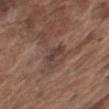notes — no biopsy performed (imaged during a skin exam) | diameter — ≈3 mm | subject — male, in their mid- to late 70s | tile lighting — white-light illumination | image source — ~15 mm tile from a whole-body skin photo | location — the arm.